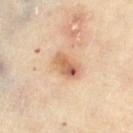Impression:
Captured during whole-body skin photography for melanoma surveillance; the lesion was not biopsied.
Acquisition and patient details:
The subject is a female aged approximately 60. A roughly 15 mm field-of-view crop from a total-body skin photograph. Longest diameter approximately 4 mm. The tile uses cross-polarized illumination. The total-body-photography lesion software estimated border irregularity of about 2 on a 0–10 scale, internal color variation of about 9 on a 0–10 scale, and peripheral color asymmetry of about 3.5. And it measured a classifier nevus-likeness of about 75/100 and a detector confidence of about 100 out of 100 that the crop contains a lesion. The lesion is on the leg.